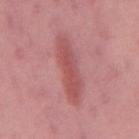No biopsy was performed on this lesion — it was imaged during a full skin examination and was not determined to be concerning.
A male subject in their mid-50s.
Cropped from a total-body skin-imaging series; the visible field is about 15 mm.
Located on the mid back.